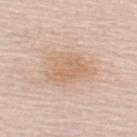Clinical impression: This lesion was catalogued during total-body skin photography and was not selected for biopsy. Acquisition and patient details: Located on the upper back. Measured at roughly 5.5 mm in maximum diameter. A male patient roughly 65 years of age. Imaged with white-light lighting. This image is a 15 mm lesion crop taken from a total-body photograph.